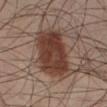Impression: No biopsy was performed on this lesion — it was imaged during a full skin examination and was not determined to be concerning. Context: The lesion is located on the leg. A 15 mm close-up extracted from a 3D total-body photography capture. A male patient about 40 years old.